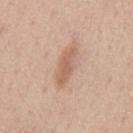biopsy status: imaged on a skin check; not biopsied
patient: male, aged approximately 60
lesion size: ~5 mm (longest diameter)
image-analysis metrics: a shape eccentricity near 0.95 and a shape-asymmetry score of about 0.3 (0 = symmetric); about 10 CIELAB-L* units darker than the surrounding skin and a lesion-to-skin contrast of about 6.5 (normalized; higher = more distinct); a nevus-likeness score of about 45/100
tile lighting: white-light
site: the mid back
imaging modality: total-body-photography crop, ~15 mm field of view